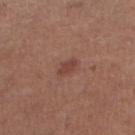* notes: imaged on a skin check; not biopsied